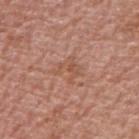{"biopsy_status": "not biopsied; imaged during a skin examination", "lesion_size": {"long_diameter_mm_approx": 3.0}, "image": {"source": "total-body photography crop", "field_of_view_mm": 15}, "site": "right upper arm", "patient": {"sex": "male", "age_approx": 70}}